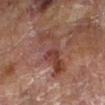Q: Was this lesion biopsied?
A: total-body-photography surveillance lesion; no biopsy
Q: What is the imaging modality?
A: 15 mm crop, total-body photography
Q: Illumination type?
A: cross-polarized
Q: Who is the patient?
A: male, in their mid-80s or older
Q: What is the lesion's diameter?
A: ≈7 mm
Q: Where on the body is the lesion?
A: the right forearm